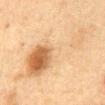  biopsy_status: not biopsied; imaged during a skin examination